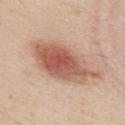Clinical impression:
Captured during whole-body skin photography for melanoma surveillance; the lesion was not biopsied.
Image and clinical context:
A male patient in their mid- to late 20s. A 15 mm close-up tile from a total-body photography series done for melanoma screening. The recorded lesion diameter is about 9 mm. On the upper back. Captured under white-light illumination.